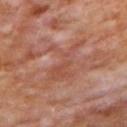Clinical impression: Part of a total-body skin-imaging series; this lesion was reviewed on a skin check and was not flagged for biopsy.